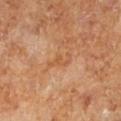Notes:
• notes · no biopsy performed (imaged during a skin exam)
• image · 15 mm crop, total-body photography
• patient · female
• TBP lesion metrics · a footprint of about 3 mm², a shape eccentricity near 0.95, and a symmetry-axis asymmetry near 0.5; an average lesion color of about L≈57 a*≈25 b*≈40 (CIELAB), a lesion–skin lightness drop of about 7, and a normalized border contrast of about 5
• lesion size · ≈3.5 mm
• body site · the left lower leg